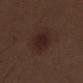Assessment:
Recorded during total-body skin imaging; not selected for excision or biopsy.
Context:
The lesion's longest dimension is about 4 mm. On the left thigh. Automated image analysis of the tile measured an eccentricity of roughly 0.55 and two-axis asymmetry of about 0.1. The analysis additionally found a border-irregularity index near 1/10, internal color variation of about 2.5 on a 0–10 scale, and peripheral color asymmetry of about 1. A male subject, in their 70s. This is a white-light tile. A region of skin cropped from a whole-body photographic capture, roughly 15 mm wide.